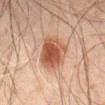Impression:
Part of a total-body skin-imaging series; this lesion was reviewed on a skin check and was not flagged for biopsy.
Context:
A male patient, aged 68 to 72. On the back. A 15 mm crop from a total-body photograph taken for skin-cancer surveillance. The lesion-visualizer software estimated an area of roughly 14 mm², an outline eccentricity of about 0.45 (0 = round, 1 = elongated), and a symmetry-axis asymmetry near 0.2. It also reported a mean CIELAB color near L≈44 a*≈21 b*≈29, a lesion–skin lightness drop of about 12, and a lesion-to-skin contrast of about 9.5 (normalized; higher = more distinct). The software also gave radial color variation of about 1.5. It also reported a nevus-likeness score of about 100/100.